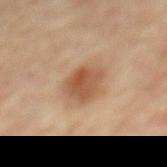| field | value |
|---|---|
| workup | imaged on a skin check; not biopsied |
| acquisition | total-body-photography crop, ~15 mm field of view |
| tile lighting | cross-polarized illumination |
| body site | the mid back |
| patient | male, in their mid-80s or older |
| size | ≈3.5 mm |